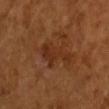follow-up — total-body-photography surveillance lesion; no biopsy
patient — female, about 55 years old
imaging modality — ~15 mm tile from a whole-body skin photo
lesion size — ~5 mm (longest diameter)
illumination — cross-polarized illumination
site — the arm
automated metrics — a lesion color around L≈32 a*≈24 b*≈33 in CIELAB, a lesion–skin lightness drop of about 7, and a normalized lesion–skin contrast near 6.5; a border-irregularity index near 6/10 and internal color variation of about 2 on a 0–10 scale